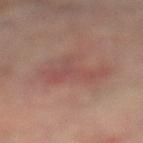Case summary:
• follow-up · catalogued during a skin exam; not biopsied
• image source · 15 mm crop, total-body photography
• lesion diameter · about 5.5 mm
• image-analysis metrics · an area of roughly 13 mm², a shape eccentricity near 0.85, and a shape-asymmetry score of about 0.55 (0 = symmetric); an average lesion color of about L≈44 a*≈20 b*≈21 (CIELAB), a lesion–skin lightness drop of about 5, and a normalized lesion–skin contrast near 4.5
• location · the right leg
• subject · female, aged 78 to 82
• tile lighting · cross-polarized illumination